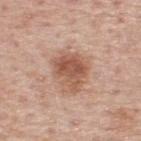Recorded during total-body skin imaging; not selected for excision or biopsy. The lesion is located on the upper back. Automated image analysis of the tile measured an area of roughly 12 mm², a shape eccentricity near 0.5, and a shape-asymmetry score of about 0.25 (0 = symmetric). And it measured a border-irregularity index near 3/10 and internal color variation of about 4.5 on a 0–10 scale. The software also gave a classifier nevus-likeness of about 70/100 and a lesion-detection confidence of about 100/100. The tile uses white-light illumination. A 15 mm close-up tile from a total-body photography series done for melanoma screening. A male patient roughly 75 years of age.